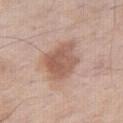No biopsy was performed on this lesion — it was imaged during a full skin examination and was not determined to be concerning.
The lesion is on the right thigh.
Imaged with white-light lighting.
A 15 mm crop from a total-body photograph taken for skin-cancer surveillance.
A male subject aged approximately 60.
Measured at roughly 5 mm in maximum diameter.